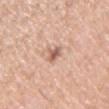site: the right upper arm | patient: male, in their mid- to late 50s | lighting: white-light | imaging modality: total-body-photography crop, ~15 mm field of view | diameter: ~2.5 mm (longest diameter).